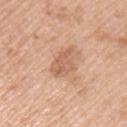Q: Was this lesion biopsied?
A: imaged on a skin check; not biopsied
Q: Automated lesion metrics?
A: an area of roughly 7 mm² and an eccentricity of roughly 0.8; roughly 9 lightness units darker than nearby skin and a normalized lesion–skin contrast near 6; an automated nevus-likeness rating near 10 out of 100
Q: How large is the lesion?
A: about 4 mm
Q: What lighting was used for the tile?
A: white-light
Q: What kind of image is this?
A: total-body-photography crop, ~15 mm field of view
Q: Who is the patient?
A: male, roughly 55 years of age
Q: Lesion location?
A: the left upper arm